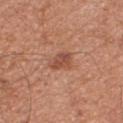The lesion was tiled from a total-body skin photograph and was not biopsied. A male subject, roughly 65 years of age. From the front of the torso. A 15 mm close-up tile from a total-body photography series done for melanoma screening.